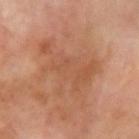biopsy status: no biopsy performed (imaged during a skin exam) | illumination: cross-polarized | image-analysis metrics: a lesion-to-skin contrast of about 5.5 (normalized; higher = more distinct); a nevus-likeness score of about 0/100 and a lesion-detection confidence of about 100/100 | subject: male, about 70 years old | anatomic site: the left upper arm | acquisition: ~15 mm crop, total-body skin-cancer survey.